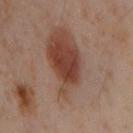<case>
  <biopsy_status>not biopsied; imaged during a skin examination</biopsy_status>
  <lesion_size>
    <long_diameter_mm_approx>3.0</long_diameter_mm_approx>
  </lesion_size>
  <image>
    <source>total-body photography crop</source>
    <field_of_view_mm>15</field_of_view_mm>
  </image>
  <patient>
    <sex>male</sex>
    <age_approx>50</age_approx>
  </patient>
  <lighting>cross-polarized</lighting>
  <site>left arm</site>
</case>